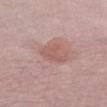The lesion was tiled from a total-body skin photograph and was not biopsied. A female patient aged approximately 60. A 15 mm crop from a total-body photograph taken for skin-cancer surveillance. Located on the chest.